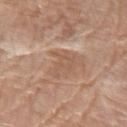A region of skin cropped from a whole-body photographic capture, roughly 15 mm wide. The lesion's longest dimension is about 3 mm. A female subject aged 63–67. The lesion is located on the left arm. Imaged with white-light lighting.